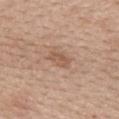| field | value |
|---|---|
| imaging modality | total-body-photography crop, ~15 mm field of view |
| automated lesion analysis | an eccentricity of roughly 0.85 and a shape-asymmetry score of about 0.25 (0 = symmetric); a lesion color around L≈55 a*≈19 b*≈29 in CIELAB, roughly 9 lightness units darker than nearby skin, and a normalized border contrast of about 6 |
| subject | female, aged approximately 50 |
| lighting | white-light |
| diameter | ≈3 mm |
| body site | the mid back |